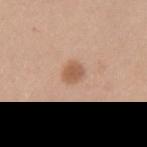Notes:
* TBP lesion metrics: a footprint of about 4.5 mm², an eccentricity of roughly 0.65, and a shape-asymmetry score of about 0.2 (0 = symmetric)
* imaging modality: ~15 mm tile from a whole-body skin photo
* subject: female, in their 40s
* site: the left upper arm
* illumination: white-light